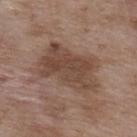Q: Is there a histopathology result?
A: no biopsy performed (imaged during a skin exam)
Q: Illumination type?
A: white-light illumination
Q: What is the anatomic site?
A: the upper back
Q: How large is the lesion?
A: ≈8 mm
Q: What kind of image is this?
A: ~15 mm tile from a whole-body skin photo
Q: Patient demographics?
A: male, about 50 years old